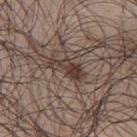The lesion was tiled from a total-body skin photograph and was not biopsied. A male patient, aged around 50. The tile uses white-light illumination. A close-up tile cropped from a whole-body skin photograph, about 15 mm across. The recorded lesion diameter is about 4.5 mm. The lesion is on the upper back.